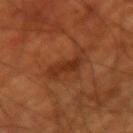Impression:
Captured during whole-body skin photography for melanoma surveillance; the lesion was not biopsied.
Acquisition and patient details:
Automated image analysis of the tile measured a lesion color around L≈32 a*≈25 b*≈33 in CIELAB, about 7 CIELAB-L* units darker than the surrounding skin, and a lesion-to-skin contrast of about 7 (normalized; higher = more distinct). The lesion's longest dimension is about 4 mm. A close-up tile cropped from a whole-body skin photograph, about 15 mm across. The patient is a male aged 68 to 72. The lesion is located on the arm. The tile uses cross-polarized illumination.